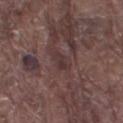workup: catalogued during a skin exam; not biopsied | image: 15 mm crop, total-body photography | automated lesion analysis: an eccentricity of roughly 0.85 and two-axis asymmetry of about 0.45; an average lesion color of about L≈33 a*≈17 b*≈16 (CIELAB) and about 6 CIELAB-L* units darker than the surrounding skin | anatomic site: the left thigh | diameter: about 2.5 mm | subject: male, aged around 80.